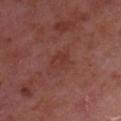TBP lesion metrics: an area of roughly 4.5 mm² and a shape eccentricity near 0.65; a mean CIELAB color near L≈37 a*≈25 b*≈26, roughly 5 lightness units darker than nearby skin, and a lesion-to-skin contrast of about 5 (normalized; higher = more distinct); a classifier nevus-likeness of about 0/100 and a detector confidence of about 100 out of 100 that the crop contains a lesion
patient: male, roughly 65 years of age
diameter: ≈3 mm
image: total-body-photography crop, ~15 mm field of view
location: the chest
tile lighting: white-light